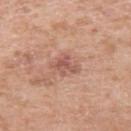| key | value |
|---|---|
| follow-up | imaged on a skin check; not biopsied |
| image | ~15 mm tile from a whole-body skin photo |
| subject | male, aged 58 to 62 |
| body site | the left upper arm |